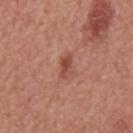{
  "biopsy_status": "not biopsied; imaged during a skin examination",
  "lesion_size": {
    "long_diameter_mm_approx": 3.0
  },
  "patient": {
    "sex": "male",
    "age_approx": 65
  },
  "lighting": "white-light",
  "image": {
    "source": "total-body photography crop",
    "field_of_view_mm": 15
  },
  "site": "back",
  "automated_metrics": {
    "cielab_L": 49,
    "cielab_a": 26,
    "cielab_b": 28,
    "vs_skin_darker_L": 9.0,
    "vs_skin_contrast_norm": 6.5
  }
}